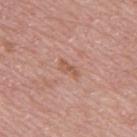{"biopsy_status": "not biopsied; imaged during a skin examination", "patient": {"sex": "male", "age_approx": 70}, "image": {"source": "total-body photography crop", "field_of_view_mm": 15}, "lesion_size": {"long_diameter_mm_approx": 3.0}, "site": "back"}